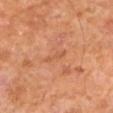| key | value |
|---|---|
| notes | total-body-photography surveillance lesion; no biopsy |
| patient | male, in their mid- to late 60s |
| location | the left upper arm |
| diameter | ~3 mm (longest diameter) |
| tile lighting | cross-polarized illumination |
| imaging modality | total-body-photography crop, ~15 mm field of view |